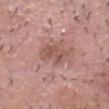Part of a total-body skin-imaging series; this lesion was reviewed on a skin check and was not flagged for biopsy.
An algorithmic analysis of the crop reported an eccentricity of roughly 0.8 and a symmetry-axis asymmetry near 0.3. It also reported an average lesion color of about L≈54 a*≈21 b*≈26 (CIELAB) and a lesion-to-skin contrast of about 6.5 (normalized; higher = more distinct).
This is a white-light tile.
The patient is a male aged approximately 55.
On the head or neck.
A 15 mm close-up tile from a total-body photography series done for melanoma screening.